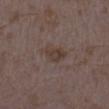  biopsy_status: not biopsied; imaged during a skin examination
  site: right lower leg
  patient:
    sex: female
    age_approx: 35
  lighting: white-light
  lesion_size:
    long_diameter_mm_approx: 4.0
  image:
    source: total-body photography crop
    field_of_view_mm: 15